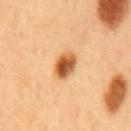notes: catalogued during a skin exam; not biopsied | image-analysis metrics: a shape eccentricity near 0.7 and a symmetry-axis asymmetry near 0.15 | patient: male, aged 48 to 52 | tile lighting: cross-polarized | size: ~3.5 mm (longest diameter) | acquisition: ~15 mm crop, total-body skin-cancer survey | anatomic site: the mid back.